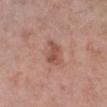Impression: The lesion was photographed on a routine skin check and not biopsied; there is no pathology result. Context: The patient is a male approximately 55 years of age. Located on the right lower leg. Cropped from a total-body skin-imaging series; the visible field is about 15 mm.